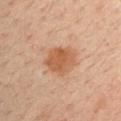The lesion was photographed on a routine skin check and not biopsied; there is no pathology result.